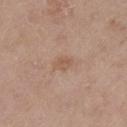Recorded during total-body skin imaging; not selected for excision or biopsy.
A male subject in their mid- to late 70s.
Imaged with white-light lighting.
Measured at roughly 2.5 mm in maximum diameter.
Cropped from a whole-body photographic skin survey; the tile spans about 15 mm.
From the left thigh.